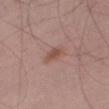The lesion was tiled from a total-body skin photograph and was not biopsied.